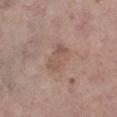Findings:
* workup · no biopsy performed (imaged during a skin exam)
* subject · female, in their mid-80s or older
* imaging modality · ~15 mm crop, total-body skin-cancer survey
* location · the right lower leg